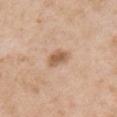Impression: This lesion was catalogued during total-body skin photography and was not selected for biopsy.